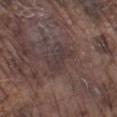Recorded during total-body skin imaging; not selected for excision or biopsy.
Measured at roughly 4 mm in maximum diameter.
The lesion is on the left lower leg.
A male subject, aged 73 to 77.
A close-up tile cropped from a whole-body skin photograph, about 15 mm across.
This is a white-light tile.